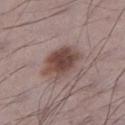Imaged during a routine full-body skin examination; the lesion was not biopsied and no histopathology is available.
From the leg.
An algorithmic analysis of the crop reported an average lesion color of about L≈45 a*≈18 b*≈21 (CIELAB).
A male patient aged approximately 70.
Cropped from a total-body skin-imaging series; the visible field is about 15 mm.
This is a white-light tile.